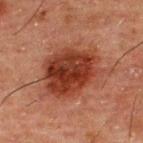The recorded lesion diameter is about 7.5 mm. The lesion is located on the upper back. Captured under cross-polarized illumination. A region of skin cropped from a whole-body photographic capture, roughly 15 mm wide. A male patient approximately 50 years of age.